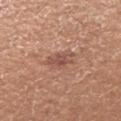Findings:
– notes — total-body-photography surveillance lesion; no biopsy
– patient — female, aged around 35
– site — the arm
– tile lighting — white-light
– image — ~15 mm tile from a whole-body skin photo